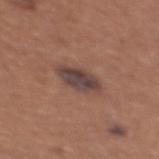<tbp_lesion>
  <biopsy_status>not biopsied; imaged during a skin examination</biopsy_status>
  <lesion_size>
    <long_diameter_mm_approx>4.5</long_diameter_mm_approx>
  </lesion_size>
  <automated_metrics>
    <cielab_L>42</cielab_L>
    <cielab_a>17</cielab_a>
    <cielab_b>20</cielab_b>
    <vs_skin_contrast_norm>10.0</vs_skin_contrast_norm>
  </automated_metrics>
  <patient>
    <sex>female</sex>
    <age_approx>35</age_approx>
  </patient>
  <site>chest</site>
  <image>
    <source>total-body photography crop</source>
    <field_of_view_mm>15</field_of_view_mm>
  </image>
</tbp_lesion>